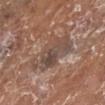The lesion was photographed on a routine skin check and not biopsied; there is no pathology result. From the left lower leg. The tile uses white-light illumination. A close-up tile cropped from a whole-body skin photograph, about 15 mm across. About 6 mm across. The patient is a female in their mid- to late 70s.